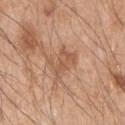Acquisition and patient details: A 15 mm close-up extracted from a 3D total-body photography capture. The total-body-photography lesion software estimated a classifier nevus-likeness of about 0/100. The lesion's longest dimension is about 4 mm. The patient is a male roughly 50 years of age. Located on the left upper arm.